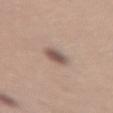Longest diameter approximately 3 mm.
The lesion is located on the left upper arm.
Imaged with white-light lighting.
A female subject in their 30s.
A 15 mm close-up extracted from a 3D total-body photography capture.